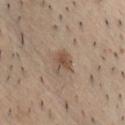Clinical impression: Captured during whole-body skin photography for melanoma surveillance; the lesion was not biopsied. Image and clinical context: Located on the chest. A male subject in their mid-30s. Captured under white-light illumination. A lesion tile, about 15 mm wide, cut from a 3D total-body photograph. The recorded lesion diameter is about 2.5 mm.